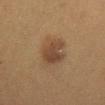No biopsy was performed on this lesion — it was imaged during a full skin examination and was not determined to be concerning.
An algorithmic analysis of the crop reported a footprint of about 11 mm², an eccentricity of roughly 0.7, and two-axis asymmetry of about 0.15. It also reported an average lesion color of about L≈36 a*≈14 b*≈26 (CIELAB), a lesion–skin lightness drop of about 8, and a normalized border contrast of about 7.
A 15 mm close-up extracted from a 3D total-body photography capture.
The recorded lesion diameter is about 4.5 mm.
Imaged with cross-polarized lighting.
A female patient about 50 years old.
From the mid back.